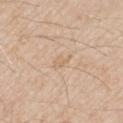Clinical impression: The lesion was tiled from a total-body skin photograph and was not biopsied. Acquisition and patient details: A 15 mm crop from a total-body photograph taken for skin-cancer surveillance. A male subject aged 63 to 67. Automated tile analysis of the lesion measured an average lesion color of about L≈66 a*≈16 b*≈34 (CIELAB). And it measured a border-irregularity index near 3.5/10 and peripheral color asymmetry of about 0.5. The software also gave a classifier nevus-likeness of about 0/100 and a detector confidence of about 100 out of 100 that the crop contains a lesion. About 2.5 mm across. This is a white-light tile. The lesion is located on the right upper arm.